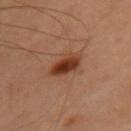The lesion was photographed on a routine skin check and not biopsied; there is no pathology result.